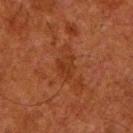Assessment: The lesion was tiled from a total-body skin photograph and was not biopsied. Background: A 15 mm close-up tile from a total-body photography series done for melanoma screening. The patient is a male in their 80s. The lesion is located on the leg.